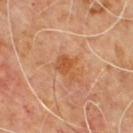workup = imaged on a skin check; not biopsied | tile lighting = cross-polarized | anatomic site = the upper back | patient = male, about 60 years old | size = ≈3.5 mm | image = 15 mm crop, total-body photography.